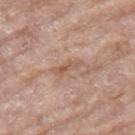Imaged during a routine full-body skin examination; the lesion was not biopsied and no histopathology is available.
The subject is a female in their mid- to late 70s.
Imaged with white-light lighting.
From the right thigh.
The recorded lesion diameter is about 3.5 mm.
A close-up tile cropped from a whole-body skin photograph, about 15 mm across.